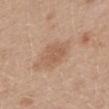Impression:
This lesion was catalogued during total-body skin photography and was not selected for biopsy.
Acquisition and patient details:
The lesion is on the mid back. A female subject, in their 40s. This is a white-light tile. Cropped from a total-body skin-imaging series; the visible field is about 15 mm. Measured at roughly 3 mm in maximum diameter.